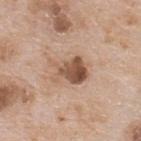Findings:
– notes — imaged on a skin check; not biopsied
– subject — male, in their mid-60s
– anatomic site — the upper back
– image — ~15 mm crop, total-body skin-cancer survey
– lesion diameter — ~4.5 mm (longest diameter)